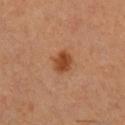| key | value |
|---|---|
| biopsy status | no biopsy performed (imaged during a skin exam) |
| size | about 2.5 mm |
| patient | female, aged 48–52 |
| lighting | cross-polarized |
| imaging modality | ~15 mm tile from a whole-body skin photo |